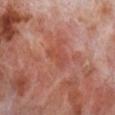  biopsy_status: not biopsied; imaged during a skin examination
  lesion_size:
    long_diameter_mm_approx: 3.0
  patient:
    sex: male
    age_approx: 70
  image:
    source: total-body photography crop
    field_of_view_mm: 15
  site: right lower leg